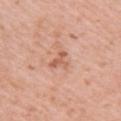The lesion was tiled from a total-body skin photograph and was not biopsied. A female subject, approximately 40 years of age. The lesion is on the right upper arm. A close-up tile cropped from a whole-body skin photograph, about 15 mm across.